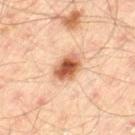Part of a total-body skin-imaging series; this lesion was reviewed on a skin check and was not flagged for biopsy.
A 15 mm crop from a total-body photograph taken for skin-cancer surveillance.
The subject is a male aged 43–47.
The lesion-visualizer software estimated an area of roughly 7.5 mm², a shape eccentricity near 0.7, and two-axis asymmetry of about 0.15. It also reported a mean CIELAB color near L≈51 a*≈22 b*≈31.
Captured under cross-polarized illumination.
Located on the left thigh.
About 3.5 mm across.